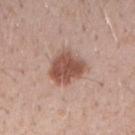Case summary:
– notes — no biopsy performed (imaged during a skin exam)